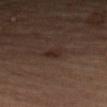biopsy status: imaged on a skin check; not biopsied
size: about 2 mm
imaging modality: 15 mm crop, total-body photography
tile lighting: cross-polarized illumination
body site: the left forearm
automated metrics: a lesion color around L≈25 a*≈16 b*≈21 in CIELAB and a normalized border contrast of about 7; border irregularity of about 2 on a 0–10 scale and a peripheral color-asymmetry measure near 0.5; a classifier nevus-likeness of about 60/100 and a lesion-detection confidence of about 100/100
patient: female, aged around 65